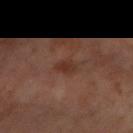This lesion was catalogued during total-body skin photography and was not selected for biopsy. The subject is a female in their 50s. A roughly 15 mm field-of-view crop from a total-body skin photograph. The lesion is located on the left forearm.